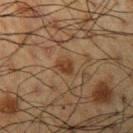Imaged during a routine full-body skin examination; the lesion was not biopsied and no histopathology is available. A male patient about 60 years old. The lesion is located on the left upper arm. Cropped from a total-body skin-imaging series; the visible field is about 15 mm. The recorded lesion diameter is about 2.5 mm. Imaged with cross-polarized lighting.